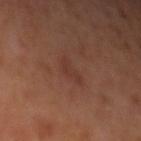Clinical impression: This lesion was catalogued during total-body skin photography and was not selected for biopsy. Acquisition and patient details: A lesion tile, about 15 mm wide, cut from a 3D total-body photograph. The subject is a female roughly 70 years of age. The lesion is on the left upper arm. About 3 mm across.